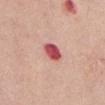<case>
<biopsy_status>not biopsied; imaged during a skin examination</biopsy_status>
<site>front of the torso</site>
<lesion_size>
  <long_diameter_mm_approx>3.0</long_diameter_mm_approx>
</lesion_size>
<patient>
  <sex>female</sex>
  <age_approx>55</age_approx>
</patient>
<image>
  <source>total-body photography crop</source>
  <field_of_view_mm>15</field_of_view_mm>
</image>
<automated_metrics>
  <area_mm2_approx>5.5</area_mm2_approx>
  <eccentricity>0.6</eccentricity>
  <shape_asymmetry>0.1</shape_asymmetry>
  <cielab_L>54</cielab_L>
  <cielab_a>36</cielab_a>
  <cielab_b>24</cielab_b>
  <vs_skin_darker_L>17.0</vs_skin_darker_L>
  <vs_skin_contrast_norm>11.0</vs_skin_contrast_norm>
  <border_irregularity_0_10>1.0</border_irregularity_0_10>
  <color_variation_0_10>4.0</color_variation_0_10>
  <peripheral_color_asymmetry>1.5</peripheral_color_asymmetry>
</automated_metrics>
<lighting>white-light</lighting>
</case>